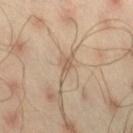Part of a total-body skin-imaging series; this lesion was reviewed on a skin check and was not flagged for biopsy.
A male subject approximately 45 years of age.
Cropped from a whole-body photographic skin survey; the tile spans about 15 mm.
The total-body-photography lesion software estimated a footprint of about 3 mm², a shape eccentricity near 0.9, and a shape-asymmetry score of about 0.5 (0 = symmetric).
From the leg.
The recorded lesion diameter is about 3.5 mm.